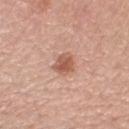Q: Was a biopsy performed?
A: no biopsy performed (imaged during a skin exam)
Q: What did automated image analysis measure?
A: an outline eccentricity of about 0.4 (0 = round, 1 = elongated) and a shape-asymmetry score of about 0.2 (0 = symmetric); a lesion color around L≈57 a*≈24 b*≈30 in CIELAB, roughly 12 lightness units darker than nearby skin, and a normalized border contrast of about 8
Q: Illumination type?
A: white-light illumination
Q: Where on the body is the lesion?
A: the leg
Q: What is the imaging modality?
A: 15 mm crop, total-body photography
Q: What are the patient's age and sex?
A: female, in their mid- to late 60s